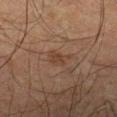workup = total-body-photography surveillance lesion; no biopsy | diameter = ≈3.5 mm | anatomic site = the left forearm | image source = ~15 mm crop, total-body skin-cancer survey | subject = male, aged approximately 65 | automated lesion analysis = an average lesion color of about L≈32 a*≈15 b*≈24 (CIELAB) and a normalized border contrast of about 6; a border-irregularity rating of about 4.5/10 and peripheral color asymmetry of about 0.5; a classifier nevus-likeness of about 10/100 and a detector confidence of about 100 out of 100 that the crop contains a lesion.